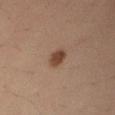The lesion was tiled from a total-body skin photograph and was not biopsied. Cropped from a total-body skin-imaging series; the visible field is about 15 mm. The lesion is located on the arm. The subject is a male approximately 35 years of age.